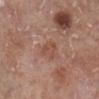Recorded during total-body skin imaging; not selected for excision or biopsy.
A female subject, approximately 75 years of age.
This image is a 15 mm lesion crop taken from a total-body photograph.
The lesion is on the left lower leg.